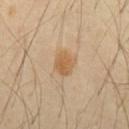Captured during whole-body skin photography for melanoma surveillance; the lesion was not biopsied.
This is a cross-polarized tile.
The patient is a male about 45 years old.
A 15 mm close-up tile from a total-body photography series done for melanoma screening.
From the abdomen.